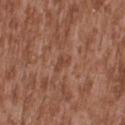Q: Was a biopsy performed?
A: imaged on a skin check; not biopsied
Q: What is the anatomic site?
A: the upper back
Q: Who is the patient?
A: male, aged approximately 45
Q: What kind of image is this?
A: ~15 mm crop, total-body skin-cancer survey
Q: What is the lesion's diameter?
A: ~2.5 mm (longest diameter)